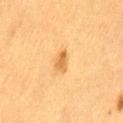The lesion was tiled from a total-body skin photograph and was not biopsied. A female patient, in their mid-50s. A close-up tile cropped from a whole-body skin photograph, about 15 mm across. Automated image analysis of the tile measured a lesion-to-skin contrast of about 7 (normalized; higher = more distinct). The software also gave a border-irregularity index near 2/10, a within-lesion color-variation index near 2/10, and peripheral color asymmetry of about 0.5. On the lower back. Approximately 2.5 mm at its widest.